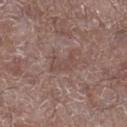Q: Was a biopsy performed?
A: catalogued during a skin exam; not biopsied
Q: Illumination type?
A: white-light illumination
Q: Lesion location?
A: the right lower leg
Q: What is the imaging modality?
A: 15 mm crop, total-body photography
Q: What is the lesion's diameter?
A: about 4 mm
Q: What did automated image analysis measure?
A: lesion-presence confidence of about 90/100
Q: Patient demographics?
A: male, aged approximately 70